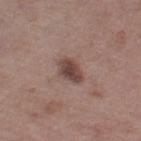No biopsy was performed on this lesion — it was imaged during a full skin examination and was not determined to be concerning.
Cropped from a total-body skin-imaging series; the visible field is about 15 mm.
Captured under white-light illumination.
About 3.5 mm across.
A female patient in their 50s.
Located on the left thigh.
The total-body-photography lesion software estimated a mean CIELAB color near L≈44 a*≈18 b*≈21, roughly 12 lightness units darker than nearby skin, and a normalized lesion–skin contrast near 9. It also reported border irregularity of about 2 on a 0–10 scale, internal color variation of about 3.5 on a 0–10 scale, and a peripheral color-asymmetry measure near 1.